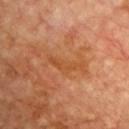* follow-up · total-body-photography surveillance lesion; no biopsy
* imaging modality · 15 mm crop, total-body photography
* patient · male, about 60 years old
* location · the front of the torso